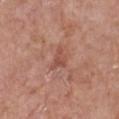Clinical impression: Recorded during total-body skin imaging; not selected for excision or biopsy. Acquisition and patient details: Automated tile analysis of the lesion measured a classifier nevus-likeness of about 0/100 and a detector confidence of about 100 out of 100 that the crop contains a lesion. Longest diameter approximately 3 mm. A female patient aged around 60. The lesion is on the chest. A lesion tile, about 15 mm wide, cut from a 3D total-body photograph.